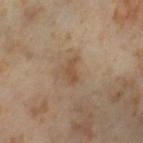<tbp_lesion>
<biopsy_status>not biopsied; imaged during a skin examination</biopsy_status>
<site>left thigh</site>
<image>
  <source>total-body photography crop</source>
  <field_of_view_mm>15</field_of_view_mm>
</image>
<patient>
  <sex>female</sex>
  <age_approx>55</age_approx>
</patient>
</tbp_lesion>